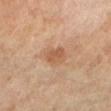Recorded during total-body skin imaging; not selected for excision or biopsy.
An algorithmic analysis of the crop reported a border-irregularity index near 2/10, a within-lesion color-variation index near 2.5/10, and peripheral color asymmetry of about 1.
From the leg.
The tile uses cross-polarized illumination.
Measured at roughly 3 mm in maximum diameter.
A female patient aged approximately 70.
This image is a 15 mm lesion crop taken from a total-body photograph.